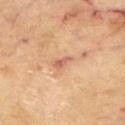Captured during whole-body skin photography for melanoma surveillance; the lesion was not biopsied.
A 15 mm close-up tile from a total-body photography series done for melanoma screening.
Imaged with cross-polarized lighting.
From the upper back.
Automated image analysis of the tile measured an average lesion color of about L≈61 a*≈24 b*≈32 (CIELAB), about 10 CIELAB-L* units darker than the surrounding skin, and a normalized border contrast of about 6.5. The software also gave an automated nevus-likeness rating near 0 out of 100.
A male subject, aged 68–72.
The recorded lesion diameter is about 3 mm.